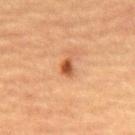notes — total-body-photography surveillance lesion; no biopsy
image — ~15 mm tile from a whole-body skin photo
diameter — ~2 mm (longest diameter)
subject — male, in their mid-60s
illumination — cross-polarized illumination
anatomic site — the back